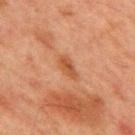biopsy status: imaged on a skin check; not biopsied
tile lighting: cross-polarized illumination
size: ~3.5 mm (longest diameter)
image-analysis metrics: a mean CIELAB color near L≈42 a*≈22 b*≈32, about 7 CIELAB-L* units darker than the surrounding skin, and a normalized border contrast of about 6.5; an automated nevus-likeness rating near 5 out of 100 and lesion-presence confidence of about 100/100
patient: male, aged 63 to 67
imaging modality: 15 mm crop, total-body photography
anatomic site: the chest